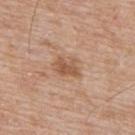{
  "biopsy_status": "not biopsied; imaged during a skin examination",
  "lighting": "white-light",
  "automated_metrics": {
    "area_mm2_approx": 5.0,
    "eccentricity": 0.75,
    "shape_asymmetry": 0.3,
    "border_irregularity_0_10": 3.5,
    "color_variation_0_10": 2.0,
    "peripheral_color_asymmetry": 0.5
  },
  "lesion_size": {
    "long_diameter_mm_approx": 3.0
  },
  "image": {
    "source": "total-body photography crop",
    "field_of_view_mm": 15
  },
  "patient": {
    "sex": "male",
    "age_approx": 65
  },
  "site": "upper back"
}